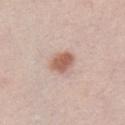Impression: This lesion was catalogued during total-body skin photography and was not selected for biopsy. Context: A male patient, aged 28–32. A lesion tile, about 15 mm wide, cut from a 3D total-body photograph.